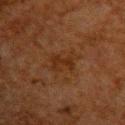Recorded during total-body skin imaging; not selected for excision or biopsy.
The lesion is located on the upper back.
About 4 mm across.
The tile uses cross-polarized illumination.
The total-body-photography lesion software estimated a footprint of about 6.5 mm² and an eccentricity of roughly 0.8. The software also gave about 5 CIELAB-L* units darker than the surrounding skin. The analysis additionally found a border-irregularity rating of about 5.5/10, a color-variation rating of about 1.5/10, and a peripheral color-asymmetry measure near 0.5.
Cropped from a whole-body photographic skin survey; the tile spans about 15 mm.
A male patient aged approximately 60.